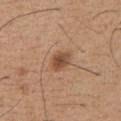Imaged during a routine full-body skin examination; the lesion was not biopsied and no histopathology is available.
From the chest.
The subject is a male in their mid- to late 60s.
A 15 mm crop from a total-body photograph taken for skin-cancer surveillance.
The lesion's longest dimension is about 3 mm.
Automated image analysis of the tile measured an area of roughly 5 mm², an outline eccentricity of about 0.6 (0 = round, 1 = elongated), and two-axis asymmetry of about 0.2. And it measured a mean CIELAB color near L≈49 a*≈21 b*≈31, roughly 12 lightness units darker than nearby skin, and a lesion-to-skin contrast of about 8.5 (normalized; higher = more distinct). The analysis additionally found a border-irregularity rating of about 1.5/10, internal color variation of about 4 on a 0–10 scale, and peripheral color asymmetry of about 1.5. The analysis additionally found a classifier nevus-likeness of about 90/100 and lesion-presence confidence of about 100/100.